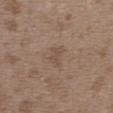Clinical impression:
Imaged during a routine full-body skin examination; the lesion was not biopsied and no histopathology is available.
Acquisition and patient details:
Automated tile analysis of the lesion measured a lesion area of about 4 mm², a shape eccentricity near 0.65, and a symmetry-axis asymmetry near 0.3. This is a white-light tile. A 15 mm crop from a total-body photograph taken for skin-cancer surveillance. Approximately 2.5 mm at its widest. A female patient, aged approximately 35. Located on the upper back.